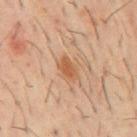The lesion is located on the mid back. Cropped from a total-body skin-imaging series; the visible field is about 15 mm. Approximately 3 mm at its widest. A male patient, aged around 60. This is a cross-polarized tile.